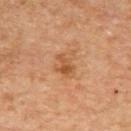Background: A female subject, aged around 60. The recorded lesion diameter is about 3 mm. On the upper back. Cropped from a total-body skin-imaging series; the visible field is about 15 mm.